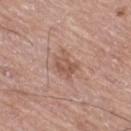The lesion was tiled from a total-body skin photograph and was not biopsied.
Longest diameter approximately 2.5 mm.
The patient is a male aged 73 to 77.
A region of skin cropped from a whole-body photographic capture, roughly 15 mm wide.
Captured under white-light illumination.
The lesion-visualizer software estimated a footprint of about 5 mm², an outline eccentricity of about 0.55 (0 = round, 1 = elongated), and a shape-asymmetry score of about 0.25 (0 = symmetric). The analysis additionally found a color-variation rating of about 3.5/10 and radial color variation of about 1.5.
On the leg.